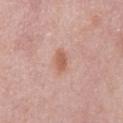Q: Is there a histopathology result?
A: no biopsy performed (imaged during a skin exam)
Q: Where on the body is the lesion?
A: the abdomen
Q: Illumination type?
A: white-light illumination
Q: How large is the lesion?
A: ≈2.5 mm
Q: What is the imaging modality?
A: ~15 mm crop, total-body skin-cancer survey
Q: Patient demographics?
A: male, in their mid- to late 50s